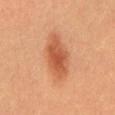follow-up: no biopsy performed (imaged during a skin exam)
image: ~15 mm crop, total-body skin-cancer survey
tile lighting: cross-polarized
patient: female, aged approximately 30
size: ≈6 mm
location: the abdomen